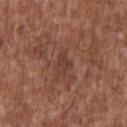Part of a total-body skin-imaging series; this lesion was reviewed on a skin check and was not flagged for biopsy. A roughly 15 mm field-of-view crop from a total-body skin photograph. This is a white-light tile. Located on the upper back. A male patient, aged approximately 45.